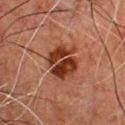| key | value |
|---|---|
| follow-up | no biopsy performed (imaged during a skin exam) |
| subject | male, aged 48 to 52 |
| diameter | ~4.5 mm (longest diameter) |
| automated lesion analysis | an average lesion color of about L≈29 a*≈22 b*≈27 (CIELAB), roughly 11 lightness units darker than nearby skin, and a lesion-to-skin contrast of about 11 (normalized; higher = more distinct); a nevus-likeness score of about 90/100 and lesion-presence confidence of about 100/100 |
| location | the front of the torso |
| acquisition | ~15 mm tile from a whole-body skin photo |